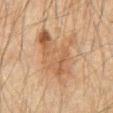{"biopsy_status": "not biopsied; imaged during a skin examination", "image": {"source": "total-body photography crop", "field_of_view_mm": 15}, "patient": {"sex": "male", "age_approx": 60}, "site": "front of the torso"}